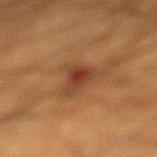A 15 mm close-up extracted from a 3D total-body photography capture.
The tile uses cross-polarized illumination.
Located on the left lower leg.
A male patient, aged 48–52.